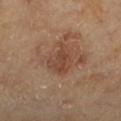<record>
  <lighting>cross-polarized</lighting>
  <site>leg</site>
  <automated_metrics>
    <color_variation_0_10>3.0</color_variation_0_10>
    <peripheral_color_asymmetry>1.0</peripheral_color_asymmetry>
    <lesion_detection_confidence_0_100>100</lesion_detection_confidence_0_100>
  </automated_metrics>
  <image>
    <source>total-body photography crop</source>
    <field_of_view_mm>15</field_of_view_mm>
  </image>
  <patient>
    <sex>female</sex>
    <age_approx>60</age_approx>
  </patient>
</record>